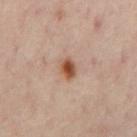Part of a total-body skin-imaging series; this lesion was reviewed on a skin check and was not flagged for biopsy.
A patient aged approximately 55.
Approximately 3 mm at its widest.
The tile uses cross-polarized illumination.
The lesion is located on the back.
A roughly 15 mm field-of-view crop from a total-body skin photograph.